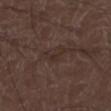| feature | finding |
|---|---|
| follow-up | total-body-photography surveillance lesion; no biopsy |
| diameter | ~3 mm (longest diameter) |
| lighting | white-light illumination |
| patient | male, aged 28 to 32 |
| anatomic site | the right lower leg |
| acquisition | ~15 mm crop, total-body skin-cancer survey |